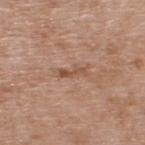Q: Was this lesion biopsied?
A: no biopsy performed (imaged during a skin exam)
Q: Lesion size?
A: ≈3 mm
Q: What is the imaging modality?
A: ~15 mm crop, total-body skin-cancer survey
Q: What is the anatomic site?
A: the upper back
Q: Patient demographics?
A: male, approximately 50 years of age